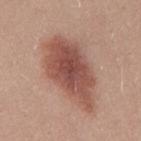The lesion was tiled from a total-body skin photograph and was not biopsied.
Captured under white-light illumination.
A male patient aged 23–27.
This image is a 15 mm lesion crop taken from a total-body photograph.
The lesion's longest dimension is about 9.5 mm.
On the mid back.